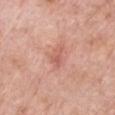follow-up = imaged on a skin check; not biopsied | image = ~15 mm tile from a whole-body skin photo | patient = male, in their 80s | site = the chest.